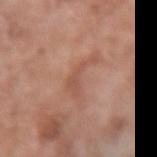Notes:
* anatomic site — the right forearm
* image source — 15 mm crop, total-body photography
* patient — female, in their mid- to late 70s
* tile lighting — white-light illumination